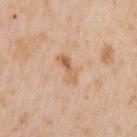Clinical impression: The lesion was tiled from a total-body skin photograph and was not biopsied. Clinical summary: The patient is a male aged 58–62. A roughly 15 mm field-of-view crop from a total-body skin photograph. The lesion is located on the left upper arm.